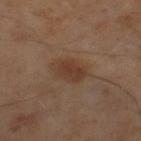The lesion was photographed on a routine skin check and not biopsied; there is no pathology result. Automated tile analysis of the lesion measured a footprint of about 7.5 mm², an eccentricity of roughly 0.8, and a shape-asymmetry score of about 0.25 (0 = symmetric). The software also gave an average lesion color of about L≈34 a*≈17 b*≈25 (CIELAB), about 7 CIELAB-L* units darker than the surrounding skin, and a lesion-to-skin contrast of about 7 (normalized; higher = more distinct). The software also gave a border-irregularity index near 2.5/10, a within-lesion color-variation index near 2/10, and radial color variation of about 0.5. The lesion's longest dimension is about 4 mm. Cropped from a total-body skin-imaging series; the visible field is about 15 mm. Located on the right lower leg. The subject is a male aged approximately 60. The tile uses cross-polarized illumination.